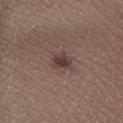biopsy status: no biopsy performed (imaged during a skin exam) | illumination: white-light | TBP lesion metrics: an outline eccentricity of about 0.65 (0 = round, 1 = elongated) and two-axis asymmetry of about 0.25; a lesion–skin lightness drop of about 10 and a normalized lesion–skin contrast near 8.5; a border-irregularity index near 2.5/10, internal color variation of about 3 on a 0–10 scale, and radial color variation of about 1; a nevus-likeness score of about 70/100 and a detector confidence of about 100 out of 100 that the crop contains a lesion | image source: total-body-photography crop, ~15 mm field of view | patient: male, in their mid- to late 20s | body site: the right lower leg.